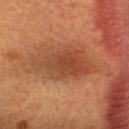workup = imaged on a skin check; not biopsied.